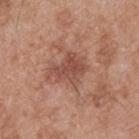No biopsy was performed on this lesion — it was imaged during a full skin examination and was not determined to be concerning. This is a white-light tile. Located on the upper back. The subject is a male in their mid-50s. Approximately 4 mm at its widest. A close-up tile cropped from a whole-body skin photograph, about 15 mm across.